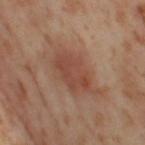No biopsy was performed on this lesion — it was imaged during a full skin examination and was not determined to be concerning.
A 15 mm close-up tile from a total-body photography series done for melanoma screening.
About 5 mm across.
The lesion is on the right thigh.
Automated tile analysis of the lesion measured a border-irregularity index near 2.5/10 and radial color variation of about 1.
A female patient, aged 53 to 57.